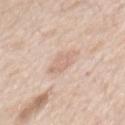notes = total-body-photography surveillance lesion; no biopsy
site = the back
lighting = white-light
patient = male, about 60 years old
TBP lesion metrics = a border-irregularity index near 3/10, a color-variation rating of about 1.5/10, and radial color variation of about 0.5; a classifier nevus-likeness of about 0/100 and a lesion-detection confidence of about 100/100
lesion diameter = ≈3.5 mm
imaging modality = 15 mm crop, total-body photography